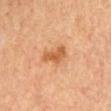  biopsy_status: not biopsied; imaged during a skin examination
  site: left upper arm
  lighting: cross-polarized
  image:
    source: total-body photography crop
    field_of_view_mm: 15
  patient:
    sex: female
    age_approx: 45